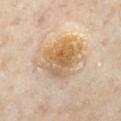Captured during whole-body skin photography for melanoma surveillance; the lesion was not biopsied.
Captured under cross-polarized illumination.
The patient is a female aged approximately 60.
The lesion is on the chest.
About 6 mm across.
The lesion-visualizer software estimated a footprint of about 21 mm² and two-axis asymmetry of about 0.3. The analysis additionally found a border-irregularity index near 4/10 and a color-variation rating of about 9.5/10. The analysis additionally found a nevus-likeness score of about 70/100.
A region of skin cropped from a whole-body photographic capture, roughly 15 mm wide.